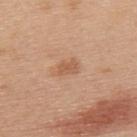Clinical impression: Captured during whole-body skin photography for melanoma surveillance; the lesion was not biopsied. Image and clinical context: The lesion's longest dimension is about 3 mm. This is a white-light tile. From the upper back. The patient is a male approximately 70 years of age. Cropped from a total-body skin-imaging series; the visible field is about 15 mm.